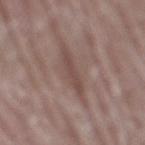No biopsy was performed on this lesion — it was imaged during a full skin examination and was not determined to be concerning. From the mid back. Imaged with white-light lighting. The subject is a male about 40 years old. A region of skin cropped from a whole-body photographic capture, roughly 15 mm wide. About 5 mm across.